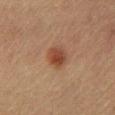Captured during whole-body skin photography for melanoma surveillance; the lesion was not biopsied.
Cropped from a total-body skin-imaging series; the visible field is about 15 mm.
Automated tile analysis of the lesion measured a shape eccentricity near 0.7 and a shape-asymmetry score of about 0.2 (0 = symmetric). The software also gave a color-variation rating of about 3.5/10 and peripheral color asymmetry of about 1.
A female subject approximately 65 years of age.
Approximately 3 mm at its widest.
On the chest.